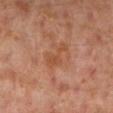Q: Is there a histopathology result?
A: imaged on a skin check; not biopsied
Q: Lesion size?
A: ~4 mm (longest diameter)
Q: What is the anatomic site?
A: the left lower leg
Q: Who is the patient?
A: male, aged 28–32
Q: How was this image acquired?
A: 15 mm crop, total-body photography